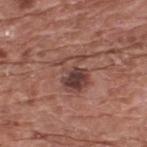This lesion was catalogued during total-body skin photography and was not selected for biopsy. This image is a 15 mm lesion crop taken from a total-body photograph. Imaged with white-light lighting. The total-body-photography lesion software estimated border irregularity of about 5.5 on a 0–10 scale, internal color variation of about 9.5 on a 0–10 scale, and peripheral color asymmetry of about 4.5. The analysis additionally found an automated nevus-likeness rating near 85 out of 100. On the upper back. Approximately 4.5 mm at its widest. A male subject, aged 73–77.